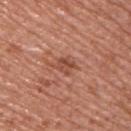Q: Was a biopsy performed?
A: imaged on a skin check; not biopsied
Q: Patient demographics?
A: male, aged 53 to 57
Q: How was this image acquired?
A: ~15 mm tile from a whole-body skin photo
Q: Lesion location?
A: the upper back